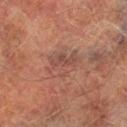No biopsy was performed on this lesion — it was imaged during a full skin examination and was not determined to be concerning. Captured under cross-polarized illumination. The lesion-visualizer software estimated a border-irregularity rating of about 4/10 and a color-variation rating of about 4/10. Longest diameter approximately 4 mm. Located on the leg. Cropped from a total-body skin-imaging series; the visible field is about 15 mm. A male subject, aged 73 to 77.